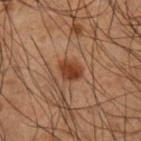  lighting: cross-polarized
  image:
    source: total-body photography crop
    field_of_view_mm: 15
  automated_metrics:
    cielab_L: 30
    cielab_a: 19
    cielab_b: 26
    vs_skin_darker_L: 9.0
    border_irregularity_0_10: 2.0
    color_variation_0_10: 2.0
    peripheral_color_asymmetry: 0.5
  site: right upper arm
  lesion_size:
    long_diameter_mm_approx: 3.0
  patient:
    sex: male
    age_approx: 50